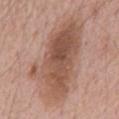A close-up tile cropped from a whole-body skin photograph, about 15 mm across. An algorithmic analysis of the crop reported a footprint of about 42 mm², an outline eccentricity of about 0.85 (0 = round, 1 = elongated), and a shape-asymmetry score of about 0.25 (0 = symmetric). It also reported internal color variation of about 6.5 on a 0–10 scale and peripheral color asymmetry of about 2.5. The analysis additionally found a nevus-likeness score of about 10/100 and a lesion-detection confidence of about 100/100. A male patient, approximately 60 years of age. Measured at roughly 10.5 mm in maximum diameter. The lesion is located on the back.